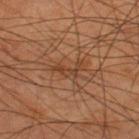Q: What is the imaging modality?
A: ~15 mm tile from a whole-body skin photo
Q: What is the anatomic site?
A: the upper back
Q: What did automated image analysis measure?
A: a mean CIELAB color near L≈40 a*≈20 b*≈31 and a lesion–skin lightness drop of about 7; border irregularity of about 7 on a 0–10 scale, internal color variation of about 2.5 on a 0–10 scale, and a peripheral color-asymmetry measure near 0.5; a classifier nevus-likeness of about 0/100 and a lesion-detection confidence of about 95/100
Q: What is the lesion's diameter?
A: ≈4 mm
Q: What lighting was used for the tile?
A: cross-polarized
Q: Who is the patient?
A: male, in their mid- to late 40s